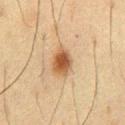This lesion was catalogued during total-body skin photography and was not selected for biopsy. On the abdomen. A roughly 15 mm field-of-view crop from a total-body skin photograph. A male patient, about 60 years old.